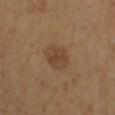{"biopsy_status": "not biopsied; imaged during a skin examination", "lesion_size": {"long_diameter_mm_approx": 3.5}, "site": "right lower leg", "image": {"source": "total-body photography crop", "field_of_view_mm": 15}, "lighting": "cross-polarized", "patient": {"sex": "female", "age_approx": 55}}